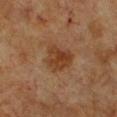biopsy status = catalogued during a skin exam; not biopsied
lighting = cross-polarized
patient = female, in their mid-50s
imaging modality = total-body-photography crop, ~15 mm field of view
anatomic site = the front of the torso